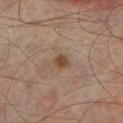No biopsy was performed on this lesion — it was imaged during a full skin examination and was not determined to be concerning. A close-up tile cropped from a whole-body skin photograph, about 15 mm across. The lesion's longest dimension is about 2 mm. Located on the leg. This is a cross-polarized tile. A male subject aged 73–77.